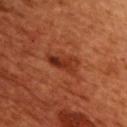Context:
The lesion-visualizer software estimated a lesion area of about 6.5 mm², an outline eccentricity of about 0.9 (0 = round, 1 = elongated), and two-axis asymmetry of about 0.35. It also reported an average lesion color of about L≈35 a*≈30 b*≈35 (CIELAB) and a lesion-to-skin contrast of about 8 (normalized; higher = more distinct). The analysis additionally found a within-lesion color-variation index near 7/10. It also reported a classifier nevus-likeness of about 5/100. The patient is a male aged 48 to 52. This image is a 15 mm lesion crop taken from a total-body photograph. Measured at roughly 4 mm in maximum diameter. The lesion is on the chest.